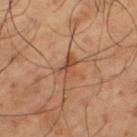biopsy status: imaged on a skin check; not biopsied
image: ~15 mm tile from a whole-body skin photo
location: the leg
subject: male, about 65 years old
lesion size: ~3 mm (longest diameter)